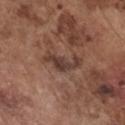biopsy status: no biopsy performed (imaged during a skin exam) | site: the chest | lesion diameter: ≈4.5 mm | tile lighting: white-light | image: total-body-photography crop, ~15 mm field of view | patient: male, in their mid- to late 70s.